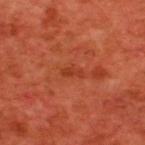The subject is a male approximately 60 years of age.
From the upper back.
A 15 mm close-up tile from a total-body photography series done for melanoma screening.